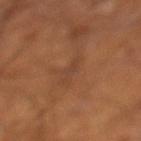workup = catalogued during a skin exam; not biopsied.